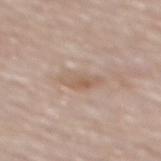The lesion was photographed on a routine skin check and not biopsied; there is no pathology result.
The lesion's longest dimension is about 4.5 mm.
A male patient, approximately 75 years of age.
The lesion is located on the mid back.
This is a white-light tile.
A 15 mm close-up extracted from a 3D total-body photography capture.